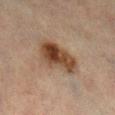* follow-up: imaged on a skin check; not biopsied
* lesion diameter: ≈5.5 mm
* imaging modality: total-body-photography crop, ~15 mm field of view
* body site: the leg
* tile lighting: cross-polarized illumination
* subject: female, aged around 70